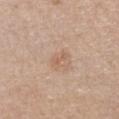Q: Was a biopsy performed?
A: imaged on a skin check; not biopsied
Q: What is the lesion's diameter?
A: about 2.5 mm
Q: Lesion location?
A: the chest
Q: What did automated image analysis measure?
A: roughly 6 lightness units darker than nearby skin and a normalized lesion–skin contrast near 5; a nevus-likeness score of about 0/100 and a lesion-detection confidence of about 100/100
Q: Who is the patient?
A: female, aged 63–67
Q: What kind of image is this?
A: ~15 mm crop, total-body skin-cancer survey
Q: What lighting was used for the tile?
A: white-light